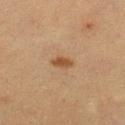Imaged during a routine full-body skin examination; the lesion was not biopsied and no histopathology is available.
A 15 mm close-up extracted from a 3D total-body photography capture.
The lesion is on the left lower leg.
About 2.5 mm across.
The subject is a female aged 38–42.
The tile uses cross-polarized illumination.